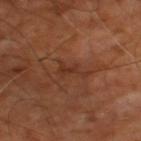Assessment: Recorded during total-body skin imaging; not selected for excision or biopsy. Clinical summary: The lesion is located on the left thigh. The lesion's longest dimension is about 3.5 mm. The subject is in their mid- to late 60s. A 15 mm close-up extracted from a 3D total-body photography capture. The tile uses cross-polarized illumination.